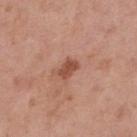Case summary:
• workup: catalogued during a skin exam; not biopsied
• anatomic site: the upper back
• image source: 15 mm crop, total-body photography
• patient: male, approximately 55 years of age
• lesion diameter: ~2.5 mm (longest diameter)
• tile lighting: white-light illumination
• image-analysis metrics: a lesion color around L≈50 a*≈25 b*≈31 in CIELAB and a lesion–skin lightness drop of about 11; border irregularity of about 2 on a 0–10 scale and peripheral color asymmetry of about 0.5; lesion-presence confidence of about 100/100